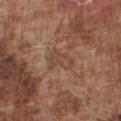Captured during whole-body skin photography for melanoma surveillance; the lesion was not biopsied. Captured under white-light illumination. On the chest. The patient is a male aged approximately 75. This image is a 15 mm lesion crop taken from a total-body photograph. An algorithmic analysis of the crop reported a footprint of about 6.5 mm² and a symmetry-axis asymmetry near 0.3.